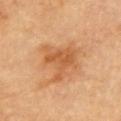Clinical summary: A male patient aged approximately 85 or older. The lesion is located on the chest. Approximately 4.5 mm at its widest. This is a cross-polarized tile. A 15 mm close-up tile from a total-body photography series done for melanoma screening.